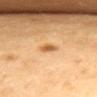A roughly 15 mm field-of-view crop from a total-body skin photograph.
A female patient, aged around 65.
The lesion is located on the chest.